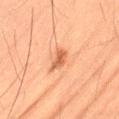| key | value |
|---|---|
| diameter | ~2.5 mm (longest diameter) |
| image | total-body-photography crop, ~15 mm field of view |
| patient | male, aged 53–57 |
| illumination | cross-polarized illumination |
| location | the right thigh |